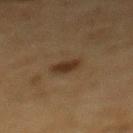Clinical impression: The lesion was photographed on a routine skin check and not biopsied; there is no pathology result.